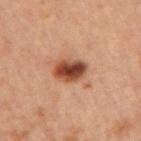Impression:
The lesion was photographed on a routine skin check and not biopsied; there is no pathology result.
Background:
A lesion tile, about 15 mm wide, cut from a 3D total-body photograph. The recorded lesion diameter is about 3.5 mm. The patient is a female approximately 45 years of age. Imaged with cross-polarized lighting. The lesion is located on the arm. Automated tile analysis of the lesion measured a normalized lesion–skin contrast near 12. And it measured a border-irregularity index near 1.5/10 and peripheral color asymmetry of about 2.5. The software also gave a classifier nevus-likeness of about 100/100 and lesion-presence confidence of about 100/100.